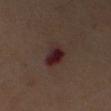Imaged during a routine full-body skin examination; the lesion was not biopsied and no histopathology is available. Located on the right upper arm. This is a cross-polarized tile. The recorded lesion diameter is about 3.5 mm. The subject is a male aged approximately 55. A 15 mm close-up tile from a total-body photography series done for melanoma screening.